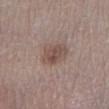• notes · catalogued during a skin exam; not biopsied
• imaging modality · 15 mm crop, total-body photography
• subject · female, approximately 65 years of age
• diameter · ≈3.5 mm
• anatomic site · the right lower leg
• tile lighting · white-light